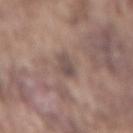Recorded during total-body skin imaging; not selected for excision or biopsy.
Measured at roughly 3 mm in maximum diameter.
A male patient, aged around 75.
This image is a 15 mm lesion crop taken from a total-body photograph.
Automated image analysis of the tile measured border irregularity of about 2 on a 0–10 scale and a within-lesion color-variation index near 2.5/10. And it measured a nevus-likeness score of about 0/100 and lesion-presence confidence of about 90/100.
The tile uses white-light illumination.
The lesion is located on the mid back.